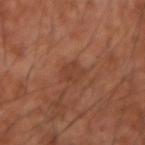{
  "biopsy_status": "not biopsied; imaged during a skin examination"
}